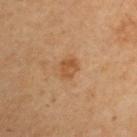The lesion was photographed on a routine skin check and not biopsied; there is no pathology result. Located on the right upper arm. The recorded lesion diameter is about 2.5 mm. A 15 mm crop from a total-body photograph taken for skin-cancer surveillance. A male patient, aged approximately 70. The tile uses cross-polarized illumination.